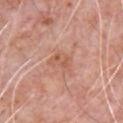Clinical summary: The lesion is located on the chest. A close-up tile cropped from a whole-body skin photograph, about 15 mm across. The total-body-photography lesion software estimated a footprint of about 5.5 mm², an eccentricity of roughly 0.7, and a symmetry-axis asymmetry near 0.4. The software also gave about 7 CIELAB-L* units darker than the surrounding skin and a normalized lesion–skin contrast near 5. It also reported a within-lesion color-variation index near 4/10. The lesion's longest dimension is about 3 mm. The patient is a male about 80 years old.